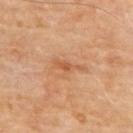| feature | finding |
|---|---|
| workup | no biopsy performed (imaged during a skin exam) |
| patient | male, roughly 70 years of age |
| acquisition | ~15 mm crop, total-body skin-cancer survey |
| anatomic site | the upper back |
| lesion size | ~4 mm (longest diameter) |